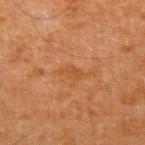No biopsy was performed on this lesion — it was imaged during a full skin examination and was not determined to be concerning. A male subject, aged 63–67. An algorithmic analysis of the crop reported a lesion area of about 3 mm² and a shape eccentricity near 0.85. The software also gave border irregularity of about 4.5 on a 0–10 scale, internal color variation of about 1 on a 0–10 scale, and peripheral color asymmetry of about 0.5. From the left upper arm. Imaged with cross-polarized lighting. A lesion tile, about 15 mm wide, cut from a 3D total-body photograph. The lesion's longest dimension is about 2.5 mm.